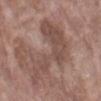Clinical impression: This lesion was catalogued during total-body skin photography and was not selected for biopsy. Context: A female patient, aged around 70. Captured under white-light illumination. The total-body-photography lesion software estimated a color-variation rating of about 3/10 and a peripheral color-asymmetry measure near 1. It also reported a classifier nevus-likeness of about 0/100 and lesion-presence confidence of about 95/100. A close-up tile cropped from a whole-body skin photograph, about 15 mm across. The lesion is on the arm. Approximately 9.5 mm at its widest.